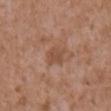| field | value |
|---|---|
| patient | male, aged approximately 75 |
| automated lesion analysis | a footprint of about 5 mm², an outline eccentricity of about 0.6 (0 = round, 1 = elongated), and a symmetry-axis asymmetry near 0.25; a lesion–skin lightness drop of about 7 and a normalized border contrast of about 6; an automated nevus-likeness rating near 0 out of 100 and a detector confidence of about 100 out of 100 that the crop contains a lesion |
| diameter | about 3 mm |
| tile lighting | white-light illumination |
| anatomic site | the abdomen |
| image | 15 mm crop, total-body photography |